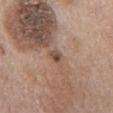This lesion was catalogued during total-body skin photography and was not selected for biopsy.
The subject is a male about 70 years old.
Longest diameter approximately 2.5 mm.
Cropped from a total-body skin-imaging series; the visible field is about 15 mm.
The lesion is on the front of the torso.